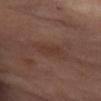| key | value |
|---|---|
| follow-up | no biopsy performed (imaged during a skin exam) |
| diameter | ≈4.5 mm |
| acquisition | ~15 mm crop, total-body skin-cancer survey |
| subject | female, aged approximately 55 |
| tile lighting | cross-polarized |
| location | the left thigh |
| image-analysis metrics | two-axis asymmetry of about 0.2; a mean CIELAB color near L≈36 a*≈19 b*≈24 and a lesion-to-skin contrast of about 5.5 (normalized; higher = more distinct); a border-irregularity index near 3.5/10, a within-lesion color-variation index near 2/10, and radial color variation of about 0.5; lesion-presence confidence of about 90/100 |